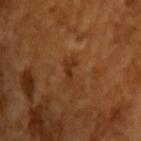Assessment: No biopsy was performed on this lesion — it was imaged during a full skin examination and was not determined to be concerning. Image and clinical context: Cropped from a total-body skin-imaging series; the visible field is about 15 mm. Measured at roughly 3 mm in maximum diameter. The lesion-visualizer software estimated a symmetry-axis asymmetry near 0.55. It also reported a mean CIELAB color near L≈31 a*≈22 b*≈33 and a normalized lesion–skin contrast near 6.5. And it measured border irregularity of about 5.5 on a 0–10 scale, a within-lesion color-variation index near 0/10, and peripheral color asymmetry of about 0. The analysis additionally found a nevus-likeness score of about 0/100 and lesion-presence confidence of about 100/100. This is a cross-polarized tile. A male subject, in their mid-60s.